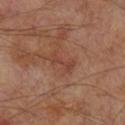Q: What are the patient's age and sex?
A: male, aged approximately 70
Q: Lesion size?
A: about 3.5 mm
Q: What is the anatomic site?
A: the right lower leg
Q: How was this image acquired?
A: ~15 mm tile from a whole-body skin photo